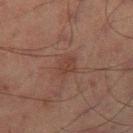Q: Was a biopsy performed?
A: catalogued during a skin exam; not biopsied
Q: What is the lesion's diameter?
A: ≈2.5 mm
Q: Lesion location?
A: the left thigh
Q: What kind of image is this?
A: ~15 mm tile from a whole-body skin photo
Q: Patient demographics?
A: male, roughly 65 years of age